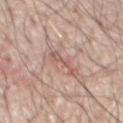biopsy status=imaged on a skin check; not biopsied | imaging modality=total-body-photography crop, ~15 mm field of view | tile lighting=white-light | diameter=≈3.5 mm | automated metrics=an average lesion color of about L≈57 a*≈21 b*≈25 (CIELAB), about 9 CIELAB-L* units darker than the surrounding skin, and a normalized lesion–skin contrast near 6; a border-irregularity rating of about 8/10, a within-lesion color-variation index near 1.5/10, and peripheral color asymmetry of about 0.5; a classifier nevus-likeness of about 0/100 and a lesion-detection confidence of about 95/100 | patient=male, about 70 years old | site=the chest.